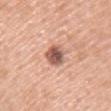• notes — no biopsy performed (imaged during a skin exam)